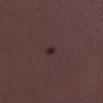Imaged during a routine full-body skin examination; the lesion was not biopsied and no histopathology is available. A lesion tile, about 15 mm wide, cut from a 3D total-body photograph. This is a white-light tile. A male patient about 30 years old. From the leg. Approximately 1.5 mm at its widest.